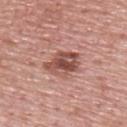No biopsy was performed on this lesion — it was imaged during a full skin examination and was not determined to be concerning.
The total-body-photography lesion software estimated a mean CIELAB color near L≈50 a*≈24 b*≈26, about 13 CIELAB-L* units darker than the surrounding skin, and a normalized lesion–skin contrast near 9. It also reported border irregularity of about 3.5 on a 0–10 scale and internal color variation of about 6.5 on a 0–10 scale. It also reported an automated nevus-likeness rating near 35 out of 100 and a lesion-detection confidence of about 100/100.
Captured under white-light illumination.
A male subject aged 73–77.
From the upper back.
Measured at roughly 4.5 mm in maximum diameter.
A 15 mm close-up tile from a total-body photography series done for melanoma screening.